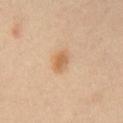follow-up = catalogued during a skin exam; not biopsied | imaging modality = ~15 mm tile from a whole-body skin photo | subject = male, in their 40s | lighting = cross-polarized | location = the abdomen.